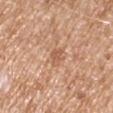| feature | finding |
|---|---|
| follow-up | catalogued during a skin exam; not biopsied |
| site | the right upper arm |
| automated metrics | an average lesion color of about L≈58 a*≈22 b*≈34 (CIELAB), roughly 8 lightness units darker than nearby skin, and a normalized lesion–skin contrast near 6; border irregularity of about 3 on a 0–10 scale and a within-lesion color-variation index near 1/10 |
| tile lighting | white-light |
| diameter | ~2.5 mm (longest diameter) |
| acquisition | ~15 mm crop, total-body skin-cancer survey |
| patient | male, in their mid-60s |